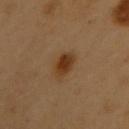– biopsy status — total-body-photography surveillance lesion; no biopsy
– subject — female, roughly 60 years of age
– image source — ~15 mm tile from a whole-body skin photo
– lesion diameter — about 3.5 mm
– location — the back
– tile lighting — cross-polarized